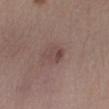Assessment: Imaged during a routine full-body skin examination; the lesion was not biopsied and no histopathology is available. Background: A 15 mm crop from a total-body photograph taken for skin-cancer surveillance. Measured at roughly 3 mm in maximum diameter. A female patient roughly 55 years of age. An algorithmic analysis of the crop reported a footprint of about 5.5 mm², a shape eccentricity near 0.6, and a shape-asymmetry score of about 0.2 (0 = symmetric). The software also gave a border-irregularity index near 2/10, a within-lesion color-variation index near 5/10, and radial color variation of about 2. The software also gave a detector confidence of about 100 out of 100 that the crop contains a lesion. On the right lower leg. The tile uses white-light illumination.